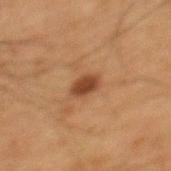{"biopsy_status": "not biopsied; imaged during a skin examination", "site": "mid back", "lesion_size": {"long_diameter_mm_approx": 3.0}, "image": {"source": "total-body photography crop", "field_of_view_mm": 15}, "patient": {"sex": "male", "age_approx": 65}}